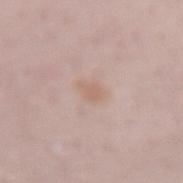biopsy_status: not biopsied; imaged during a skin examination
lighting: white-light
automated_metrics:
  cielab_L: 63
  cielab_a: 18
  cielab_b: 26
  vs_skin_darker_L: 6.0
  vs_skin_contrast_norm: 5.0
  border_irregularity_0_10: 2.5
  color_variation_0_10: 1.0
  peripheral_color_asymmetry: 0.5
  nevus_likeness_0_100: 10
image:
  source: total-body photography crop
  field_of_view_mm: 15
patient:
  sex: male
  age_approx: 50
site: left forearm